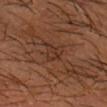Q: Where on the body is the lesion?
A: the head or neck
Q: What is the imaging modality?
A: ~15 mm tile from a whole-body skin photo
Q: Patient demographics?
A: male, aged 38–42
Q: What lighting was used for the tile?
A: cross-polarized